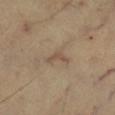<case>
<biopsy_status>not biopsied; imaged during a skin examination</biopsy_status>
<lighting>cross-polarized</lighting>
<patient>
  <age_approx>60</age_approx>
</patient>
<site>right lower leg</site>
<image>
  <source>total-body photography crop</source>
  <field_of_view_mm>15</field_of_view_mm>
</image>
</case>